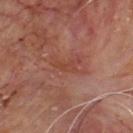<record>
<biopsy_status>not biopsied; imaged during a skin examination</biopsy_status>
<image>
  <source>total-body photography crop</source>
  <field_of_view_mm>15</field_of_view_mm>
</image>
<patient>
  <sex>male</sex>
  <age_approx>60</age_approx>
</patient>
<automated_metrics>
  <eccentricity>0.9</eccentricity>
  <shape_asymmetry>0.35</shape_asymmetry>
  <border_irregularity_0_10>4.0</border_irregularity_0_10>
  <color_variation_0_10>0.0</color_variation_0_10>
  <peripheral_color_asymmetry>0.0</peripheral_color_asymmetry>
  <nevus_likeness_0_100>0</nevus_likeness_0_100>
  <lesion_detection_confidence_0_100>75</lesion_detection_confidence_0_100>
</automated_metrics>
<site>back</site>
</record>